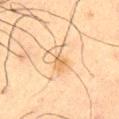notes = total-body-photography surveillance lesion; no biopsy | body site = the abdomen | imaging modality = total-body-photography crop, ~15 mm field of view | lighting = cross-polarized | lesion diameter = ~3.5 mm (longest diameter) | patient = male, aged 58 to 62.